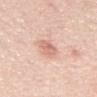Assessment:
Imaged during a routine full-body skin examination; the lesion was not biopsied and no histopathology is available.
Context:
Imaged with white-light lighting. An algorithmic analysis of the crop reported a nevus-likeness score of about 45/100 and a detector confidence of about 100 out of 100 that the crop contains a lesion. A 15 mm close-up extracted from a 3D total-body photography capture. A male patient in their 70s. The lesion is on the mid back.